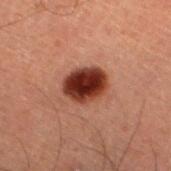Imaged during a routine full-body skin examination; the lesion was not biopsied and no histopathology is available.
The patient is a male in their 60s.
This is a cross-polarized tile.
On the left lower leg.
Approximately 4.5 mm at its widest.
Cropped from a total-body skin-imaging series; the visible field is about 15 mm.